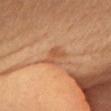Case summary:
- notes · imaged on a skin check; not biopsied
- body site · the head or neck
- diameter · ~3 mm (longest diameter)
- patient · female, roughly 70 years of age
- image · ~15 mm crop, total-body skin-cancer survey
- lighting · cross-polarized